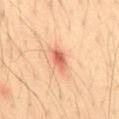Assessment:
The lesion was photographed on a routine skin check and not biopsied; there is no pathology result.
Background:
The total-body-photography lesion software estimated a lesion area of about 5 mm² and an eccentricity of roughly 0.8. The analysis additionally found a detector confidence of about 100 out of 100 that the crop contains a lesion. A male patient roughly 35 years of age. A 15 mm crop from a total-body photograph taken for skin-cancer surveillance. From the lower back. Longest diameter approximately 3 mm. This is a cross-polarized tile.